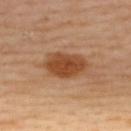Captured during whole-body skin photography for melanoma surveillance; the lesion was not biopsied.
A female subject aged 38–42.
This image is a 15 mm lesion crop taken from a total-body photograph.
Located on the back.
Captured under cross-polarized illumination.
Measured at roughly 5.5 mm in maximum diameter.